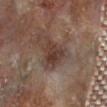Assessment:
Captured during whole-body skin photography for melanoma surveillance; the lesion was not biopsied.
Clinical summary:
A male subject, approximately 85 years of age or older. The lesion is located on the right forearm. Cropped from a whole-body photographic skin survey; the tile spans about 15 mm.